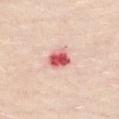Findings:
- TBP lesion metrics · a lesion area of about 5.5 mm² and an outline eccentricity of about 0.6 (0 = round, 1 = elongated)
- subject · female, aged 53–57
- tile lighting · white-light illumination
- site · the chest
- lesion diameter · ~3 mm (longest diameter)
- image · total-body-photography crop, ~15 mm field of view